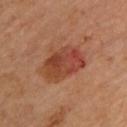The lesion was photographed on a routine skin check and not biopsied; there is no pathology result.
A female patient approximately 70 years of age.
Located on the upper back.
A 15 mm close-up extracted from a 3D total-body photography capture.